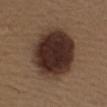Case summary:
- image source · ~15 mm crop, total-body skin-cancer survey
- subject · female, aged 38–42
- illumination · white-light illumination
- automated metrics · a border-irregularity index near 1/10 and radial color variation of about 1.5
- site · the upper back
- lesion diameter · ≈7 mm